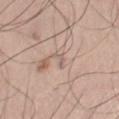Impression: This lesion was catalogued during total-body skin photography and was not selected for biopsy.